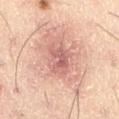Findings:
– follow-up — no biopsy performed (imaged during a skin exam)
– acquisition — ~15 mm tile from a whole-body skin photo
– diameter — about 4 mm
– lighting — cross-polarized
– subject — male, about 50 years old
– anatomic site — the left thigh